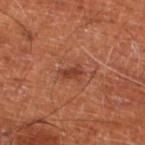follow-up — imaged on a skin check; not biopsied
lesion size — ≈2.5 mm
acquisition — 15 mm crop, total-body photography
anatomic site — the left lower leg
patient — in their mid-60s
tile lighting — cross-polarized illumination